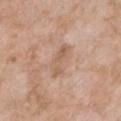Assessment: The lesion was photographed on a routine skin check and not biopsied; there is no pathology result. Background: Cropped from a total-body skin-imaging series; the visible field is about 15 mm. The subject is a female in their mid-70s. The lesion is located on the front of the torso.